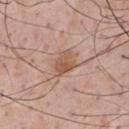Impression: The lesion was photographed on a routine skin check and not biopsied; there is no pathology result. Background: Imaged with white-light lighting. Approximately 2.5 mm at its widest. The lesion is located on the chest. A 15 mm close-up extracted from a 3D total-body photography capture. The subject is a male aged 53–57.